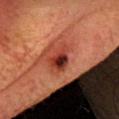Assessment: Captured during whole-body skin photography for melanoma surveillance; the lesion was not biopsied. Clinical summary: Measured at roughly 3 mm in maximum diameter. The tile uses cross-polarized illumination. A male subject, approximately 70 years of age. A roughly 15 mm field-of-view crop from a total-body skin photograph. Located on the head or neck.